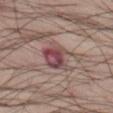{
  "image": {
    "source": "total-body photography crop",
    "field_of_view_mm": 15
  },
  "patient": {
    "sex": "male",
    "age_approx": 55
  },
  "lighting": "white-light",
  "automated_metrics": {
    "shape_asymmetry": 0.3,
    "cielab_L": 47,
    "cielab_a": 22,
    "cielab_b": 18,
    "vs_skin_darker_L": 12.0,
    "vs_skin_contrast_norm": 9.0,
    "border_irregularity_0_10": 3.5,
    "color_variation_0_10": 9.0,
    "peripheral_color_asymmetry": 3.0,
    "nevus_likeness_0_100": 0
  },
  "lesion_size": {
    "long_diameter_mm_approx": 4.5
  },
  "site": "left thigh"
}